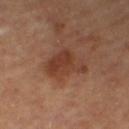workup: catalogued during a skin exam; not biopsied
illumination: cross-polarized
acquisition: ~15 mm tile from a whole-body skin photo
body site: the right thigh
size: ~5.5 mm (longest diameter)
subject: female, aged 58–62